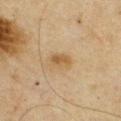Notes:
• follow-up · catalogued during a skin exam; not biopsied
• location · the chest
• tile lighting · cross-polarized
• image-analysis metrics · a lesion area of about 4 mm², an outline eccentricity of about 0.75 (0 = round, 1 = elongated), and two-axis asymmetry of about 0.25; internal color variation of about 2 on a 0–10 scale and a peripheral color-asymmetry measure near 0.5; an automated nevus-likeness rating near 30 out of 100 and a detector confidence of about 100 out of 100 that the crop contains a lesion
• diameter · ~2.5 mm (longest diameter)
• acquisition · total-body-photography crop, ~15 mm field of view
• subject · male, approximately 65 years of age